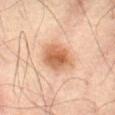<record>
  <biopsy_status>not biopsied; imaged during a skin examination</biopsy_status>
</record>